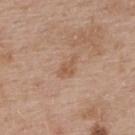Case summary:
• lesion size · about 3 mm
• acquisition · ~15 mm crop, total-body skin-cancer survey
• subject · female, about 40 years old
• illumination · white-light illumination
• site · the upper back